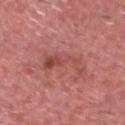Case summary:
- follow-up — total-body-photography surveillance lesion; no biopsy
- diameter — ≈6 mm
- image source — ~15 mm tile from a whole-body skin photo
- lighting — white-light
- anatomic site — the head or neck
- subject — male, aged 73 to 77